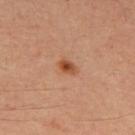Impression: The lesion was photographed on a routine skin check and not biopsied; there is no pathology result. Acquisition and patient details: From the upper back. This is a cross-polarized tile. A male subject in their mid-60s. This image is a 15 mm lesion crop taken from a total-body photograph. Approximately 2.5 mm at its widest.